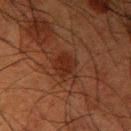Case summary:
– biopsy status: no biopsy performed (imaged during a skin exam)